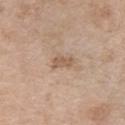| key | value |
|---|---|
| follow-up | catalogued during a skin exam; not biopsied |
| subject | female, aged 73–77 |
| tile lighting | white-light illumination |
| automated lesion analysis | a border-irregularity index near 2.5/10 and radial color variation of about 0.5; a classifier nevus-likeness of about 0/100 and a detector confidence of about 100 out of 100 that the crop contains a lesion |
| lesion size | about 2.5 mm |
| acquisition | ~15 mm tile from a whole-body skin photo |
| location | the left upper arm |